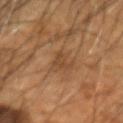{
  "biopsy_status": "not biopsied; imaged during a skin examination",
  "site": "head or neck",
  "patient": {
    "sex": "male",
    "age_approx": 55
  },
  "image": {
    "source": "total-body photography crop",
    "field_of_view_mm": 15
  }
}